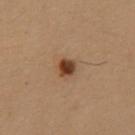Assessment:
Captured during whole-body skin photography for melanoma surveillance; the lesion was not biopsied.
Clinical summary:
Automated tile analysis of the lesion measured a footprint of about 4 mm² and an eccentricity of roughly 0.65. And it measured border irregularity of about 2 on a 0–10 scale, internal color variation of about 4.5 on a 0–10 scale, and a peripheral color-asymmetry measure near 1.5. It also reported a lesion-detection confidence of about 100/100. The subject is a female roughly 50 years of age. About 2.5 mm across. The lesion is on the chest. This image is a 15 mm lesion crop taken from a total-body photograph.